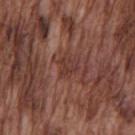<record>
<biopsy_status>not biopsied; imaged during a skin examination</biopsy_status>
<automated_metrics>
  <vs_skin_darker_L>6.0</vs_skin_darker_L>
  <vs_skin_contrast_norm>5.5</vs_skin_contrast_norm>
  <border_irregularity_0_10>4.0</border_irregularity_0_10>
  <peripheral_color_asymmetry>1.0</peripheral_color_asymmetry>
  <lesion_detection_confidence_0_100>75</lesion_detection_confidence_0_100>
</automated_metrics>
<patient>
  <sex>male</sex>
  <age_approx>75</age_approx>
</patient>
<site>chest</site>
<image>
  <source>total-body photography crop</source>
  <field_of_view_mm>15</field_of_view_mm>
</image>
</record>